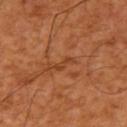The lesion was photographed on a routine skin check and not biopsied; there is no pathology result.
A 15 mm close-up extracted from a 3D total-body photography capture.
On the right upper arm.
The tile uses cross-polarized illumination.
A patient approximately 65 years of age.
Automated image analysis of the tile measured a lesion area of about 2.5 mm², an eccentricity of roughly 0.9, and a shape-asymmetry score of about 0.4 (0 = symmetric). And it measured a peripheral color-asymmetry measure near 0.